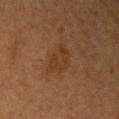Located on the arm. The lesion's longest dimension is about 4.5 mm. A region of skin cropped from a whole-body photographic capture, roughly 15 mm wide. Imaged with cross-polarized lighting. A female subject aged around 50.